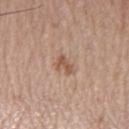Q: Was this lesion biopsied?
A: catalogued during a skin exam; not biopsied
Q: Who is the patient?
A: male, roughly 60 years of age
Q: What is the imaging modality?
A: total-body-photography crop, ~15 mm field of view
Q: Lesion location?
A: the left forearm